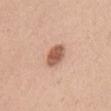Assessment:
This lesion was catalogued during total-body skin photography and was not selected for biopsy.
Background:
Imaged with white-light lighting. Located on the chest. A male subject about 40 years old. A region of skin cropped from a whole-body photographic capture, roughly 15 mm wide.